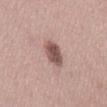<lesion>
<biopsy_status>not biopsied; imaged during a skin examination</biopsy_status>
<site>left thigh</site>
<lighting>white-light</lighting>
<image>
  <source>total-body photography crop</source>
  <field_of_view_mm>15</field_of_view_mm>
</image>
<lesion_size>
  <long_diameter_mm_approx>3.0</long_diameter_mm_approx>
</lesion_size>
<patient>
  <sex>female</sex>
  <age_approx>45</age_approx>
</patient>
</lesion>